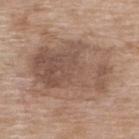  biopsy_status: not biopsied; imaged during a skin examination
  patient:
    sex: female
    age_approx: 75
  image:
    source: total-body photography crop
    field_of_view_mm: 15
  site: upper back
  lesion_size:
    long_diameter_mm_approx: 9.5
  automated_metrics:
    border_irregularity_0_10: 2.5
    color_variation_0_10: 6.5
    nevus_likeness_0_100: 25
    lesion_detection_confidence_0_100: 100
  lighting: white-light